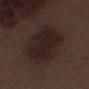<case>
  <biopsy_status>not biopsied; imaged during a skin examination</biopsy_status>
  <site>right thigh</site>
  <patient>
    <sex>male</sex>
    <age_approx>70</age_approx>
  </patient>
  <image>
    <source>total-body photography crop</source>
    <field_of_view_mm>15</field_of_view_mm>
  </image>
</case>